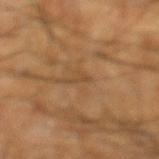Imaged during a routine full-body skin examination; the lesion was not biopsied and no histopathology is available.
Automated image analysis of the tile measured a footprint of about 1.5 mm². It also reported a lesion color around L≈45 a*≈17 b*≈34 in CIELAB, about 6 CIELAB-L* units darker than the surrounding skin, and a normalized lesion–skin contrast near 5. The analysis additionally found an automated nevus-likeness rating near 0 out of 100 and lesion-presence confidence of about 55/100.
This image is a 15 mm lesion crop taken from a total-body photograph.
Imaged with cross-polarized lighting.
A male patient aged approximately 60.
From the left lower leg.
Longest diameter approximately 2.5 mm.